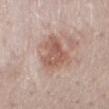biopsy status: catalogued during a skin exam; not biopsied
image: ~15 mm tile from a whole-body skin photo
patient: female, aged approximately 50
tile lighting: white-light
automated lesion analysis: an outline eccentricity of about 0.55 (0 = round, 1 = elongated) and two-axis asymmetry of about 0.4; a classifier nevus-likeness of about 20/100
body site: the mid back